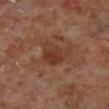Located on the right lower leg. A male patient, about 60 years old. Captured under cross-polarized illumination. Cropped from a total-body skin-imaging series; the visible field is about 15 mm.